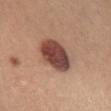No biopsy was performed on this lesion — it was imaged during a full skin examination and was not determined to be concerning. Imaged with white-light lighting. A female patient about 35 years old. A 15 mm close-up tile from a total-body photography series done for melanoma screening. Located on the chest.